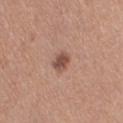No biopsy was performed on this lesion — it was imaged during a full skin examination and was not determined to be concerning. The total-body-photography lesion software estimated an average lesion color of about L≈50 a*≈21 b*≈26 (CIELAB) and roughly 12 lightness units darker than nearby skin. The software also gave a color-variation rating of about 2/10. The software also gave a nevus-likeness score of about 70/100 and a lesion-detection confidence of about 100/100. Cropped from a total-body skin-imaging series; the visible field is about 15 mm. From the right thigh. Approximately 2.5 mm at its widest. Captured under white-light illumination. The patient is a female aged approximately 50.